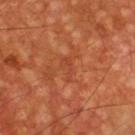Clinical impression: Imaged during a routine full-body skin examination; the lesion was not biopsied and no histopathology is available. Background: A 15 mm close-up tile from a total-body photography series done for melanoma screening. The lesion is on the chest. A male patient, roughly 60 years of age.